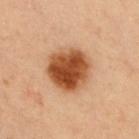Impression: This lesion was catalogued during total-body skin photography and was not selected for biopsy. Background: The subject is a female aged 38–42. The tile uses cross-polarized illumination. On the chest. A 15 mm close-up extracted from a 3D total-body photography capture. The lesion's longest dimension is about 5 mm. The lesion-visualizer software estimated an average lesion color of about L≈41 a*≈21 b*≈32 (CIELAB), roughly 15 lightness units darker than nearby skin, and a normalized lesion–skin contrast near 12. The analysis additionally found a border-irregularity rating of about 1/10, internal color variation of about 6 on a 0–10 scale, and radial color variation of about 2.